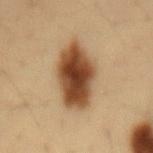{
  "biopsy_status": "not biopsied; imaged during a skin examination",
  "image": {
    "source": "total-body photography crop",
    "field_of_view_mm": 15
  },
  "site": "back",
  "patient": {
    "sex": "male",
    "age_approx": 35
  },
  "automated_metrics": {
    "shape_asymmetry": 0.15,
    "cielab_L": 42,
    "cielab_a": 19,
    "cielab_b": 32,
    "vs_skin_contrast_norm": 13.5,
    "border_irregularity_0_10": 2.0,
    "color_variation_0_10": 6.5,
    "peripheral_color_asymmetry": 2.0,
    "nevus_likeness_0_100": 100,
    "lesion_detection_confidence_0_100": 100
  }
}